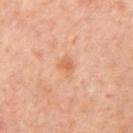biopsy status: total-body-photography surveillance lesion; no biopsy | tile lighting: cross-polarized illumination | lesion diameter: ~2.5 mm (longest diameter) | subject: female, about 50 years old | automated metrics: border irregularity of about 2.5 on a 0–10 scale and a peripheral color-asymmetry measure near 0.5 | acquisition: ~15 mm tile from a whole-body skin photo | body site: the right upper arm.